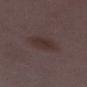Notes:
• notes · total-body-photography surveillance lesion; no biopsy
• tile lighting · white-light
• patient · female, aged 28–32
• anatomic site · the left lower leg
• lesion diameter · ~3.5 mm (longest diameter)
• acquisition · 15 mm crop, total-body photography